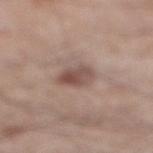Findings:
• biopsy status — no biopsy performed (imaged during a skin exam)
• body site — the right lower leg
• lesion size — ~3.5 mm (longest diameter)
• patient — male, aged around 55
• tile lighting — white-light
• acquisition — ~15 mm tile from a whole-body skin photo